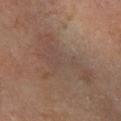Assessment: Imaged during a routine full-body skin examination; the lesion was not biopsied and no histopathology is available. Background: The lesion is located on the right lower leg. Approximately 9 mm at its widest. A male subject in their mid-60s. A lesion tile, about 15 mm wide, cut from a 3D total-body photograph. The tile uses cross-polarized illumination.